A roughly 15 mm field-of-view crop from a total-body skin photograph · a male subject, aged 48–52 · the lesion is located on the mid back:
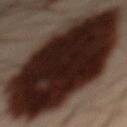Histopathologically confirmed as an indeterminate (borderline) lesion: atypical melanocytic neoplasm.